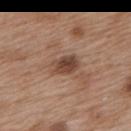Imaged during a routine full-body skin examination; the lesion was not biopsied and no histopathology is available. The lesion is located on the upper back. This is a white-light tile. A roughly 15 mm field-of-view crop from a total-body skin photograph. The subject is a female aged around 40.